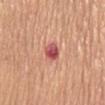Findings:
– notes · imaged on a skin check; not biopsied
– lighting · white-light
– automated lesion analysis · a lesion area of about 4.5 mm² and a shape-asymmetry score of about 0.25 (0 = symmetric); a lesion color around L≈54 a*≈33 b*≈26 in CIELAB and a normalized lesion–skin contrast near 10
– acquisition · ~15 mm crop, total-body skin-cancer survey
– lesion diameter · about 2.5 mm
– site · the front of the torso
– subject · female, roughly 65 years of age